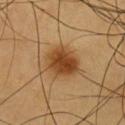The lesion was photographed on a routine skin check and not biopsied; there is no pathology result.
The total-body-photography lesion software estimated a mean CIELAB color near L≈44 a*≈22 b*≈38, about 13 CIELAB-L* units darker than the surrounding skin, and a lesion-to-skin contrast of about 10 (normalized; higher = more distinct). The software also gave border irregularity of about 1.5 on a 0–10 scale, a within-lesion color-variation index near 4.5/10, and a peripheral color-asymmetry measure near 1.5. The software also gave an automated nevus-likeness rating near 100 out of 100 and lesion-presence confidence of about 100/100.
On the chest.
A close-up tile cropped from a whole-body skin photograph, about 15 mm across.
Approximately 4 mm at its widest.
The subject is a male roughly 60 years of age.
Captured under cross-polarized illumination.